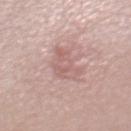Clinical impression: Recorded during total-body skin imaging; not selected for excision or biopsy. Acquisition and patient details: A female patient, about 40 years old. A region of skin cropped from a whole-body photographic capture, roughly 15 mm wide. Measured at roughly 5.5 mm in maximum diameter. This is a white-light tile. The lesion is on the chest.